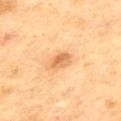Captured during whole-body skin photography for melanoma surveillance; the lesion was not biopsied. Imaged with cross-polarized lighting. The lesion's longest dimension is about 2.5 mm. The lesion is on the back. The subject is a male approximately 55 years of age. Automated image analysis of the tile measured a footprint of about 4 mm², a shape eccentricity near 0.7, and a shape-asymmetry score of about 0.2 (0 = symmetric). The software also gave an average lesion color of about L≈64 a*≈23 b*≈42 (CIELAB), roughly 11 lightness units darker than nearby skin, and a lesion-to-skin contrast of about 7 (normalized; higher = more distinct). The software also gave an automated nevus-likeness rating near 85 out of 100 and a detector confidence of about 100 out of 100 that the crop contains a lesion. A 15 mm close-up tile from a total-body photography series done for melanoma screening.